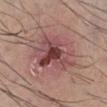workup: imaged on a skin check; not biopsied | illumination: white-light | lesion diameter: about 7 mm | image-analysis metrics: an area of roughly 21 mm², an outline eccentricity of about 0.75 (0 = round, 1 = elongated), and a shape-asymmetry score of about 0.25 (0 = symmetric); a lesion color around L≈46 a*≈24 b*≈20 in CIELAB and a lesion–skin lightness drop of about 12; border irregularity of about 3.5 on a 0–10 scale and a within-lesion color-variation index near 8.5/10; an automated nevus-likeness rating near 0 out of 100 and lesion-presence confidence of about 100/100 | subject: male, in their mid-50s | image source: total-body-photography crop, ~15 mm field of view.